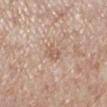{
  "biopsy_status": "not biopsied; imaged during a skin examination",
  "image": {
    "source": "total-body photography crop",
    "field_of_view_mm": 15
  },
  "patient": {
    "sex": "female",
    "age_approx": 70
  },
  "lesion_size": {
    "long_diameter_mm_approx": 2.5
  },
  "automated_metrics": {
    "cielab_L": 60,
    "cielab_a": 18,
    "cielab_b": 28,
    "vs_skin_darker_L": 8.0,
    "vs_skin_contrast_norm": 5.5,
    "peripheral_color_asymmetry": 1.0
  },
  "site": "left lower leg",
  "lighting": "white-light"
}